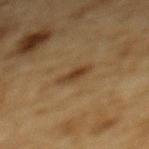{"patient": {"sex": "male", "age_approx": "85+"}, "site": "mid back", "image": {"source": "total-body photography crop", "field_of_view_mm": 15}, "lesion_size": {"long_diameter_mm_approx": 3.0}}